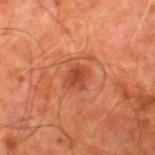Q: Was this lesion biopsied?
A: no biopsy performed (imaged during a skin exam)
Q: Patient demographics?
A: male, roughly 80 years of age
Q: Automated lesion metrics?
A: an outline eccentricity of about 0.6 (0 = round, 1 = elongated); a mean CIELAB color near L≈37 a*≈27 b*≈30, a lesion–skin lightness drop of about 8, and a normalized border contrast of about 6.5; border irregularity of about 2 on a 0–10 scale, a within-lesion color-variation index near 3.5/10, and radial color variation of about 1.5
Q: Lesion location?
A: the right thigh
Q: How was the tile lit?
A: cross-polarized illumination
Q: How large is the lesion?
A: ≈2.5 mm
Q: What kind of image is this?
A: 15 mm crop, total-body photography